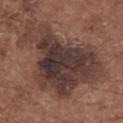{"biopsy_status": "not biopsied; imaged during a skin examination", "patient": {"sex": "female", "age_approx": 75}, "image": {"source": "total-body photography crop", "field_of_view_mm": 15}, "lesion_size": {"long_diameter_mm_approx": 11.0}, "site": "upper back", "automated_metrics": {"area_mm2_approx": 50.0, "shape_asymmetry": 0.5, "vs_skin_contrast_norm": 11.0, "border_irregularity_0_10": 6.5, "color_variation_0_10": 6.5, "peripheral_color_asymmetry": 1.5}}